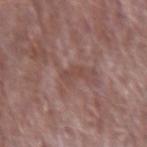location: the arm; patient: male, aged 63–67; automated metrics: border irregularity of about 3.5 on a 0–10 scale, internal color variation of about 0 on a 0–10 scale, and radial color variation of about 0; acquisition: ~15 mm crop, total-body skin-cancer survey; diameter: ≈2.5 mm; illumination: white-light.